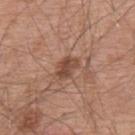Imaged during a routine full-body skin examination; the lesion was not biopsied and no histopathology is available.
A 15 mm close-up extracted from a 3D total-body photography capture.
Imaged with white-light lighting.
A male subject roughly 55 years of age.
On the upper back.